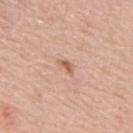notes: imaged on a skin check; not biopsied
image source: total-body-photography crop, ~15 mm field of view
tile lighting: white-light illumination
subject: male, aged 68 to 72
lesion diameter: about 2.5 mm
automated metrics: a lesion area of about 2.5 mm² and a symmetry-axis asymmetry near 0.3; a lesion color around L≈60 a*≈22 b*≈31 in CIELAB, about 10 CIELAB-L* units darker than the surrounding skin, and a lesion-to-skin contrast of about 7 (normalized; higher = more distinct)
anatomic site: the upper back